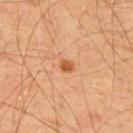notes = no biopsy performed (imaged during a skin exam)
body site = the back
illumination = cross-polarized
image source = 15 mm crop, total-body photography
subject = male, about 55 years old
size = about 1.5 mm